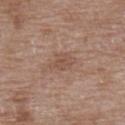Q: Is there a histopathology result?
A: no biopsy performed (imaged during a skin exam)
Q: What is the anatomic site?
A: the upper back
Q: What lighting was used for the tile?
A: white-light illumination
Q: Lesion size?
A: about 3 mm
Q: What are the patient's age and sex?
A: female, in their 70s
Q: What is the imaging modality?
A: 15 mm crop, total-body photography